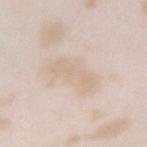Impression: Recorded during total-body skin imaging; not selected for excision or biopsy. Clinical summary: On the right forearm. Longest diameter approximately 5 mm. The patient is a female aged around 25. Imaged with white-light lighting. A close-up tile cropped from a whole-body skin photograph, about 15 mm across.